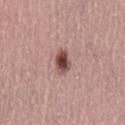Located on the lower back.
The patient is a female aged around 50.
A close-up tile cropped from a whole-body skin photograph, about 15 mm across.